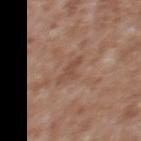Captured during whole-body skin photography for melanoma surveillance; the lesion was not biopsied.
Automated tile analysis of the lesion measured a mean CIELAB color near L≈48 a*≈19 b*≈28 and a normalized lesion–skin contrast near 5.5. The software also gave border irregularity of about 4 on a 0–10 scale, a within-lesion color-variation index near 1/10, and a peripheral color-asymmetry measure near 0.5.
This is a white-light tile.
A male patient, in their mid-40s.
The lesion is located on the upper back.
This image is a 15 mm lesion crop taken from a total-body photograph.